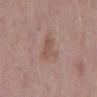Clinical summary:
The patient is a male aged 68 to 72. From the mid back. An algorithmic analysis of the crop reported a footprint of about 6.5 mm², a shape eccentricity near 0.6, and a symmetry-axis asymmetry near 0.4. It also reported a lesion color around L≈53 a*≈19 b*≈25 in CIELAB, about 7 CIELAB-L* units darker than the surrounding skin, and a normalized lesion–skin contrast near 5.5. The analysis additionally found lesion-presence confidence of about 100/100. This image is a 15 mm lesion crop taken from a total-body photograph.